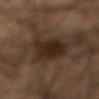Part of a total-body skin-imaging series; this lesion was reviewed on a skin check and was not flagged for biopsy. The lesion-visualizer software estimated an eccentricity of roughly 0.95 and two-axis asymmetry of about 0.3. The software also gave an average lesion color of about L≈23 a*≈14 b*≈20 (CIELAB), a lesion–skin lightness drop of about 10, and a normalized border contrast of about 11. The recorded lesion diameter is about 8.5 mm. A male subject, roughly 55 years of age. The lesion is on the abdomen. Cropped from a whole-body photographic skin survey; the tile spans about 15 mm. The tile uses cross-polarized illumination.